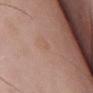biopsy status: imaged on a skin check; not biopsied | image source: 15 mm crop, total-body photography | tile lighting: white-light illumination | site: the chest | lesion size: about 1.5 mm | subject: male, in their mid- to late 50s.